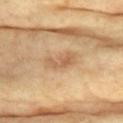No biopsy was performed on this lesion — it was imaged during a full skin examination and was not determined to be concerning.
The patient is a female aged 73–77.
The lesion is located on the chest.
A region of skin cropped from a whole-body photographic capture, roughly 15 mm wide.
Measured at roughly 3.5 mm in maximum diameter.
Captured under cross-polarized illumination.
Automated image analysis of the tile measured a footprint of about 5.5 mm² and a shape eccentricity near 0.85. It also reported a lesion color around L≈60 a*≈19 b*≈37 in CIELAB, about 9 CIELAB-L* units darker than the surrounding skin, and a normalized lesion–skin contrast near 5.5.